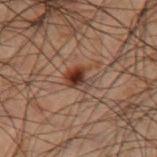workup — catalogued during a skin exam; not biopsied | TBP lesion metrics — internal color variation of about 9.5 on a 0–10 scale and peripheral color asymmetry of about 3 | patient — male, roughly 50 years of age | imaging modality — ~15 mm crop, total-body skin-cancer survey | tile lighting — cross-polarized | lesion size — about 3 mm | body site — the right thigh.